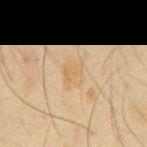  biopsy_status: not biopsied; imaged during a skin examination
  site: chest
  lesion_size:
    long_diameter_mm_approx: 3.0
  image:
    source: total-body photography crop
    field_of_view_mm: 15
  patient:
    sex: male
    age_approx: 65
  automated_metrics:
    area_mm2_approx: 5.5
    eccentricity: 0.6
    shape_asymmetry: 0.25
    border_irregularity_0_10: 3.0
    color_variation_0_10: 2.0
    peripheral_color_asymmetry: 0.5
    lesion_detection_confidence_0_100: 100